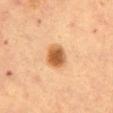workup: no biopsy performed (imaged during a skin exam)
diameter: about 3.5 mm
tile lighting: cross-polarized illumination
image: ~15 mm tile from a whole-body skin photo
patient: female, in their 60s
location: the front of the torso